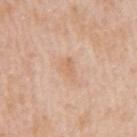follow-up: total-body-photography surveillance lesion; no biopsy
lighting: white-light
diameter: ~3 mm (longest diameter)
subject: female, aged approximately 55
acquisition: ~15 mm tile from a whole-body skin photo
body site: the right upper arm